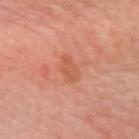Part of a total-body skin-imaging series; this lesion was reviewed on a skin check and was not flagged for biopsy.
A region of skin cropped from a whole-body photographic capture, roughly 15 mm wide.
Longest diameter approximately 2.5 mm.
The lesion is located on the left forearm.
The subject is a female aged around 40.